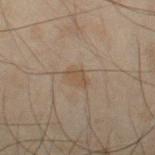The lesion was photographed on a routine skin check and not biopsied; there is no pathology result. The patient is a male aged around 50. Cropped from a total-body skin-imaging series; the visible field is about 15 mm. From the left forearm.